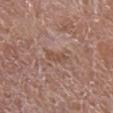| field | value |
|---|---|
| biopsy status | imaged on a skin check; not biopsied |
| illumination | white-light illumination |
| image | 15 mm crop, total-body photography |
| patient | female, aged around 80 |
| body site | the right lower leg |
| automated metrics | an average lesion color of about L≈50 a*≈20 b*≈26 (CIELAB), a lesion–skin lightness drop of about 7, and a normalized border contrast of about 6 |